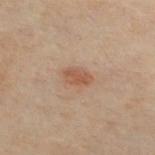Background: Longest diameter approximately 3 mm. The tile uses cross-polarized illumination. Located on the front of the torso. A close-up tile cropped from a whole-body skin photograph, about 15 mm across. A male patient about 50 years old. The lesion-visualizer software estimated an area of roughly 4 mm² and a shape eccentricity near 0.85.